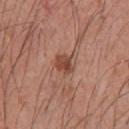{"biopsy_status": "not biopsied; imaged during a skin examination", "image": {"source": "total-body photography crop", "field_of_view_mm": 15}, "patient": {"sex": "male", "age_approx": 45}, "site": "upper back"}